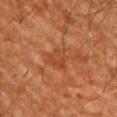Recorded during total-body skin imaging; not selected for excision or biopsy. A male patient aged around 60. Located on the left upper arm. A 15 mm crop from a total-body photograph taken for skin-cancer surveillance.